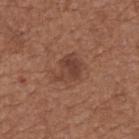Case summary:
– notes · no biopsy performed (imaged during a skin exam)
– imaging modality · ~15 mm tile from a whole-body skin photo
– location · the upper back
– automated metrics · a footprint of about 8 mm², an outline eccentricity of about 0.65 (0 = round, 1 = elongated), and two-axis asymmetry of about 0.35; an average lesion color of about L≈41 a*≈21 b*≈27 (CIELAB) and a normalized lesion–skin contrast near 6.5; a detector confidence of about 100 out of 100 that the crop contains a lesion
– subject · female, in their mid-60s
– tile lighting · white-light